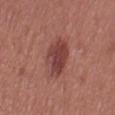notes = total-body-photography surveillance lesion; no biopsy
location = the leg
image = ~15 mm tile from a whole-body skin photo
subject = female, aged around 40